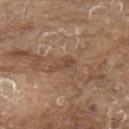notes: catalogued during a skin exam; not biopsied